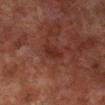This lesion was catalogued during total-body skin photography and was not selected for biopsy.
From the leg.
Approximately 3 mm at its widest.
A 15 mm crop from a total-body photograph taken for skin-cancer surveillance.
An algorithmic analysis of the crop reported an outline eccentricity of about 0.8 (0 = round, 1 = elongated) and a shape-asymmetry score of about 0.35 (0 = symmetric). It also reported an average lesion color of about L≈23 a*≈21 b*≈21 (CIELAB), a lesion–skin lightness drop of about 5, and a normalized border contrast of about 6.5. The software also gave an automated nevus-likeness rating near 0 out of 100 and lesion-presence confidence of about 100/100.
A male subject, approximately 70 years of age.